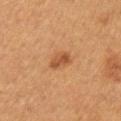<lesion>
  <automated_metrics>
    <area_mm2_approx>3.5</area_mm2_approx>
    <eccentricity>0.85</eccentricity>
    <shape_asymmetry>0.25</shape_asymmetry>
    <cielab_L>41</cielab_L>
    <cielab_a>21</cielab_a>
    <cielab_b>32</cielab_b>
    <vs_skin_darker_L>9.0</vs_skin_darker_L>
    <vs_skin_contrast_norm>7.5</vs_skin_contrast_norm>
    <nevus_likeness_0_100>90</nevus_likeness_0_100>
  </automated_metrics>
  <lesion_size>
    <long_diameter_mm_approx>3.0</long_diameter_mm_approx>
  </lesion_size>
  <image>
    <source>total-body photography crop</source>
    <field_of_view_mm>15</field_of_view_mm>
  </image>
  <site>left upper arm</site>
  <patient>
    <sex>female</sex>
    <age_approx>40</age_approx>
  </patient>
  <lighting>cross-polarized</lighting>
</lesion>